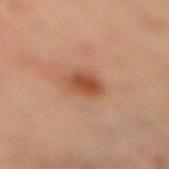– follow-up: total-body-photography surveillance lesion; no biopsy
– tile lighting: cross-polarized illumination
– acquisition: ~15 mm crop, total-body skin-cancer survey
– diameter: ~3 mm (longest diameter)
– image-analysis metrics: a lesion area of about 6.5 mm², an outline eccentricity of about 0.6 (0 = round, 1 = elongated), and a symmetry-axis asymmetry near 0.25; a border-irregularity index near 2/10, a within-lesion color-variation index near 2.5/10, and a peripheral color-asymmetry measure near 0.5
– body site: the right lower leg
– subject: female, in their 70s